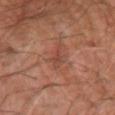Case summary:
* subject: approximately 65 years of age
* tile lighting: cross-polarized illumination
* acquisition: ~15 mm crop, total-body skin-cancer survey
* lesion size: about 4 mm
* image-analysis metrics: a mean CIELAB color near L≈44 a*≈24 b*≈28, roughly 7 lightness units darker than nearby skin, and a normalized lesion–skin contrast near 5.5
* site: the left forearm